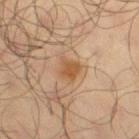• biopsy status: total-body-photography surveillance lesion; no biopsy
• acquisition: ~15 mm tile from a whole-body skin photo
• location: the right thigh
• subject: male, in their mid-60s
• lighting: cross-polarized